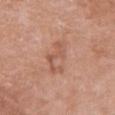Impression:
No biopsy was performed on this lesion — it was imaged during a full skin examination and was not determined to be concerning.